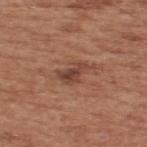Impression:
The lesion was tiled from a total-body skin photograph and was not biopsied.
Clinical summary:
An algorithmic analysis of the crop reported an average lesion color of about L≈43 a*≈22 b*≈27 (CIELAB), roughly 10 lightness units darker than nearby skin, and a normalized border contrast of about 8. And it measured border irregularity of about 6 on a 0–10 scale, internal color variation of about 2.5 on a 0–10 scale, and a peripheral color-asymmetry measure near 1. It also reported a classifier nevus-likeness of about 35/100 and a detector confidence of about 100 out of 100 that the crop contains a lesion. The subject is a male aged 53–57. About 4 mm across. The lesion is located on the upper back. A region of skin cropped from a whole-body photographic capture, roughly 15 mm wide. Captured under white-light illumination.